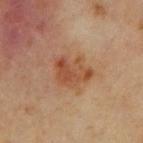Part of a total-body skin-imaging series; this lesion was reviewed on a skin check and was not flagged for biopsy.
Located on the abdomen.
Automated image analysis of the tile measured a lesion area of about 10 mm², an eccentricity of roughly 0.7, and a symmetry-axis asymmetry near 0.4. The analysis additionally found an average lesion color of about L≈47 a*≈23 b*≈32 (CIELAB), a lesion–skin lightness drop of about 9, and a lesion-to-skin contrast of about 7 (normalized; higher = more distinct). It also reported a border-irregularity index near 4.5/10 and peripheral color asymmetry of about 2. The analysis additionally found a classifier nevus-likeness of about 25/100 and a lesion-detection confidence of about 100/100.
Approximately 4.5 mm at its widest.
The subject is a male roughly 65 years of age.
Captured under cross-polarized illumination.
This image is a 15 mm lesion crop taken from a total-body photograph.